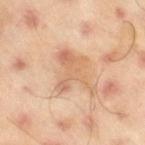Clinical impression:
The lesion was tiled from a total-body skin photograph and was not biopsied.
Clinical summary:
Captured under cross-polarized illumination. A lesion tile, about 15 mm wide, cut from a 3D total-body photograph. From the leg. A male patient aged 43 to 47. About 5.5 mm across.